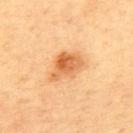Captured during whole-body skin photography for melanoma surveillance; the lesion was not biopsied. The tile uses cross-polarized illumination. A lesion tile, about 15 mm wide, cut from a 3D total-body photograph. A male subject, aged approximately 60. Located on the back.